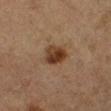Image and clinical context: About 3 mm across. Imaged with cross-polarized lighting. A 15 mm close-up extracted from a 3D total-body photography capture. Located on the left lower leg. A male patient aged around 85 or older.